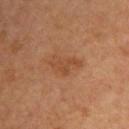{
  "automated_metrics": {
    "area_mm2_approx": 7.0,
    "eccentricity": 0.8,
    "shape_asymmetry": 0.45,
    "cielab_L": 45,
    "cielab_a": 22,
    "cielab_b": 33,
    "vs_skin_darker_L": 6.0,
    "vs_skin_contrast_norm": 5.5
  },
  "lighting": "cross-polarized",
  "image": {
    "source": "total-body photography crop",
    "field_of_view_mm": 15
  },
  "lesion_size": {
    "long_diameter_mm_approx": 4.0
  },
  "patient": {
    "sex": "male",
    "age_approx": 45
  },
  "site": "upper back"
}